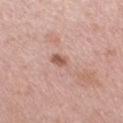Recorded during total-body skin imaging; not selected for excision or biopsy. The total-body-photography lesion software estimated an area of roughly 4.5 mm², an outline eccentricity of about 0.7 (0 = round, 1 = elongated), and two-axis asymmetry of about 0.3. It also reported a classifier nevus-likeness of about 5/100 and a lesion-detection confidence of about 100/100. A roughly 15 mm field-of-view crop from a total-body skin photograph. On the right thigh. Measured at roughly 3 mm in maximum diameter. The tile uses white-light illumination. A female patient in their mid- to late 50s.